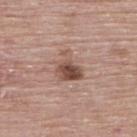Captured during whole-body skin photography for melanoma surveillance; the lesion was not biopsied.
This image is a 15 mm lesion crop taken from a total-body photograph.
The patient is a male aged 83 or older.
The lesion is on the upper back.
The recorded lesion diameter is about 4 mm.
This is a white-light tile.